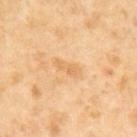Assessment:
Imaged during a routine full-body skin examination; the lesion was not biopsied and no histopathology is available.
Clinical summary:
A male subject, about 65 years old. Cropped from a total-body skin-imaging series; the visible field is about 15 mm.